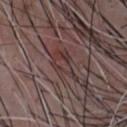Q: Was this lesion biopsied?
A: catalogued during a skin exam; not biopsied
Q: How was this image acquired?
A: ~15 mm tile from a whole-body skin photo
Q: What are the patient's age and sex?
A: male, aged 48–52
Q: What is the anatomic site?
A: the chest